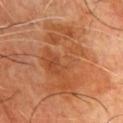follow-up: total-body-photography surveillance lesion; no biopsy | acquisition: total-body-photography crop, ~15 mm field of view | illumination: cross-polarized illumination | location: the chest | image-analysis metrics: border irregularity of about 7 on a 0–10 scale and internal color variation of about 4.5 on a 0–10 scale; an automated nevus-likeness rating near 0 out of 100 and a detector confidence of about 100 out of 100 that the crop contains a lesion | patient: male, roughly 60 years of age.